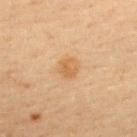Impression: The lesion was tiled from a total-body skin photograph and was not biopsied. Context: Approximately 2.5 mm at its widest. The patient is a male roughly 40 years of age. Located on the upper back. A lesion tile, about 15 mm wide, cut from a 3D total-body photograph.